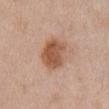subject = female, roughly 50 years of age
body site = the front of the torso
acquisition = 15 mm crop, total-body photography
lighting = white-light
lesion size = ~4 mm (longest diameter)